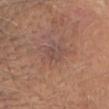This lesion was catalogued during total-body skin photography and was not selected for biopsy.
On the head or neck.
A close-up tile cropped from a whole-body skin photograph, about 15 mm across.
The subject is a male roughly 85 years of age or older.
Imaged with white-light lighting.